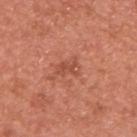{
  "biopsy_status": "not biopsied; imaged during a skin examination"
}